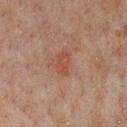| feature | finding |
|---|---|
| size | ≈3 mm |
| anatomic site | the chest |
| illumination | cross-polarized |
| acquisition | ~15 mm tile from a whole-body skin photo |
| patient | male, aged approximately 60 |